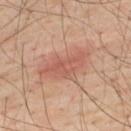Assessment: Part of a total-body skin-imaging series; this lesion was reviewed on a skin check and was not flagged for biopsy. Clinical summary: About 7 mm across. Located on the upper back. The tile uses cross-polarized illumination. A close-up tile cropped from a whole-body skin photograph, about 15 mm across. A male subject, aged approximately 40. An algorithmic analysis of the crop reported an average lesion color of about L≈58 a*≈24 b*≈29 (CIELAB) and a lesion-to-skin contrast of about 5.5 (normalized; higher = more distinct). It also reported an automated nevus-likeness rating near 85 out of 100 and a detector confidence of about 100 out of 100 that the crop contains a lesion.